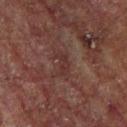notes: catalogued during a skin exam; not biopsied | lesion diameter: about 2.5 mm | body site: the chest | illumination: cross-polarized | patient: male, aged around 55 | imaging modality: total-body-photography crop, ~15 mm field of view | image-analysis metrics: an outline eccentricity of about 0.85 (0 = round, 1 = elongated) and a symmetry-axis asymmetry near 0.55; an average lesion color of about L≈28 a*≈19 b*≈19 (CIELAB) and a normalized lesion–skin contrast near 5.5; peripheral color asymmetry of about 0.